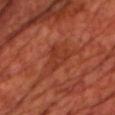Clinical impression:
Imaged during a routine full-body skin examination; the lesion was not biopsied and no histopathology is available.
Background:
The subject is a male aged 68–72. The lesion-visualizer software estimated a footprint of about 6.5 mm², an outline eccentricity of about 0.6 (0 = round, 1 = elongated), and a shape-asymmetry score of about 0.45 (0 = symmetric). The software also gave a lesion color around L≈36 a*≈30 b*≈32 in CIELAB and a normalized lesion–skin contrast near 5.5. It also reported border irregularity of about 5 on a 0–10 scale and a peripheral color-asymmetry measure near 1. Imaged with cross-polarized lighting. Longest diameter approximately 3.5 mm. The lesion is located on the chest. A lesion tile, about 15 mm wide, cut from a 3D total-body photograph.